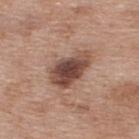The lesion was tiled from a total-body skin photograph and was not biopsied.